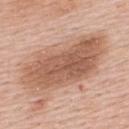A 15 mm close-up extracted from a 3D total-body photography capture. On the back. A male patient in their mid-50s.